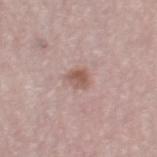Notes:
• notes: catalogued during a skin exam; not biopsied
• lighting: white-light illumination
• body site: the right thigh
• image source: 15 mm crop, total-body photography
• patient: male, about 55 years old